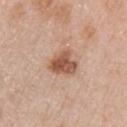Clinical summary:
Measured at roughly 3.5 mm in maximum diameter. From the right upper arm. This is a white-light tile. A female subject about 65 years old. A close-up tile cropped from a whole-body skin photograph, about 15 mm across. The lesion-visualizer software estimated a lesion area of about 7.5 mm², an outline eccentricity of about 0.5 (0 = round, 1 = elongated), and a shape-asymmetry score of about 0.35 (0 = symmetric). The software also gave border irregularity of about 3 on a 0–10 scale, internal color variation of about 4 on a 0–10 scale, and peripheral color asymmetry of about 1.5. The analysis additionally found an automated nevus-likeness rating near 70 out of 100 and a detector confidence of about 100 out of 100 that the crop contains a lesion.